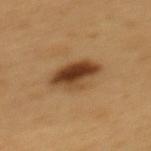Findings:
* biopsy status · no biopsy performed (imaged during a skin exam)
* image · 15 mm crop, total-body photography
* image-analysis metrics · a border-irregularity index near 2.5/10 and internal color variation of about 8 on a 0–10 scale; a nevus-likeness score of about 95/100 and a lesion-detection confidence of about 100/100
* lesion size · ≈5 mm
* tile lighting · cross-polarized
* site · the mid back
* subject · male, roughly 60 years of age